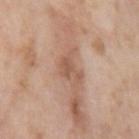Imaged during a routine full-body skin examination; the lesion was not biopsied and no histopathology is available. A close-up tile cropped from a whole-body skin photograph, about 15 mm across. From the left upper arm. Measured at roughly 3 mm in maximum diameter. The tile uses white-light illumination. Automated image analysis of the tile measured a footprint of about 5 mm², a shape eccentricity near 0.8, and a symmetry-axis asymmetry near 0.45. And it measured border irregularity of about 5.5 on a 0–10 scale and a within-lesion color-variation index near 1.5/10. The patient is a male in their 60s.